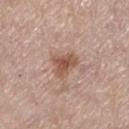Impression: Imaged during a routine full-body skin examination; the lesion was not biopsied and no histopathology is available. Image and clinical context: On the left lower leg. A female subject about 60 years old. The recorded lesion diameter is about 3.5 mm. A 15 mm crop from a total-body photograph taken for skin-cancer surveillance.